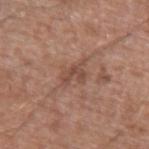The lesion was tiled from a total-body skin photograph and was not biopsied. The lesion is on the left upper arm. The subject is a male in their mid- to late 50s. About 3.5 mm across. The total-body-photography lesion software estimated an area of roughly 4.5 mm², an eccentricity of roughly 0.75, and a shape-asymmetry score of about 0.55 (0 = symmetric). And it measured roughly 8 lightness units darker than nearby skin and a normalized border contrast of about 6. And it measured a color-variation rating of about 1.5/10 and radial color variation of about 0.5. It also reported a nevus-likeness score of about 0/100. Captured under white-light illumination. A region of skin cropped from a whole-body photographic capture, roughly 15 mm wide.